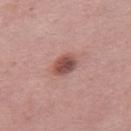Imaged during a routine full-body skin examination; the lesion was not biopsied and no histopathology is available. The lesion is located on the right thigh. A 15 mm close-up extracted from a 3D total-body photography capture. A female subject roughly 40 years of age.